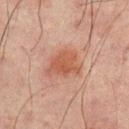Captured during whole-body skin photography for melanoma surveillance; the lesion was not biopsied. Cropped from a total-body skin-imaging series; the visible field is about 15 mm. The lesion's longest dimension is about 4 mm. The total-body-photography lesion software estimated a footprint of about 9 mm², an outline eccentricity of about 0.7 (0 = round, 1 = elongated), and two-axis asymmetry of about 0.35. It also reported an average lesion color of about L≈43 a*≈21 b*≈26 (CIELAB), about 8 CIELAB-L* units darker than the surrounding skin, and a normalized border contrast of about 7. The software also gave a classifier nevus-likeness of about 85/100 and a detector confidence of about 100 out of 100 that the crop contains a lesion. From the mid back. A male subject approximately 65 years of age.